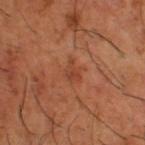- lesion diameter — about 3 mm
- tile lighting — cross-polarized illumination
- subject — male, approximately 65 years of age
- anatomic site — the right thigh
- automated lesion analysis — a lesion area of about 3.5 mm², an eccentricity of roughly 0.75, and two-axis asymmetry of about 0.45; a border-irregularity rating of about 4.5/10, a within-lesion color-variation index near 2.5/10, and peripheral color asymmetry of about 0.5
- image source — ~15 mm crop, total-body skin-cancer survey